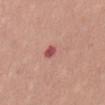Impression:
No biopsy was performed on this lesion — it was imaged during a full skin examination and was not determined to be concerning.
Clinical summary:
The lesion is located on the abdomen. A female subject, aged around 35. A lesion tile, about 15 mm wide, cut from a 3D total-body photograph.